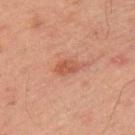{
  "biopsy_status": "not biopsied; imaged during a skin examination",
  "patient": {
    "sex": "male",
    "age_approx": 45
  },
  "image": {
    "source": "total-body photography crop",
    "field_of_view_mm": 15
  },
  "site": "left upper arm",
  "lighting": "cross-polarized"
}